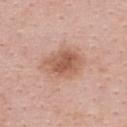follow-up: catalogued during a skin exam; not biopsied
automated metrics: a shape-asymmetry score of about 0.2 (0 = symmetric); a lesion color around L≈59 a*≈22 b*≈30 in CIELAB, roughly 11 lightness units darker than nearby skin, and a lesion-to-skin contrast of about 7.5 (normalized; higher = more distinct); a border-irregularity rating of about 2/10, internal color variation of about 4.5 on a 0–10 scale, and peripheral color asymmetry of about 1.5; a lesion-detection confidence of about 100/100
tile lighting: white-light illumination
location: the upper back
diameter: about 5.5 mm
subject: female, approximately 35 years of age
image source: ~15 mm crop, total-body skin-cancer survey